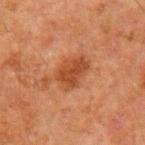Impression: This lesion was catalogued during total-body skin photography and was not selected for biopsy. Acquisition and patient details: From the left upper arm. This is a cross-polarized tile. A male patient aged approximately 60. A 15 mm crop from a total-body photograph taken for skin-cancer surveillance.